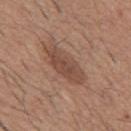Acquisition and patient details:
Automated image analysis of the tile measured a footprint of about 12 mm² and two-axis asymmetry of about 0.15. The analysis additionally found an automated nevus-likeness rating near 45 out of 100 and a detector confidence of about 100 out of 100 that the crop contains a lesion. The tile uses white-light illumination. The subject is a male aged 58 to 62. A 15 mm close-up extracted from a 3D total-body photography capture. Measured at roughly 6 mm in maximum diameter. The lesion is on the mid back.